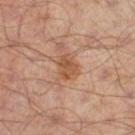Clinical impression:
Imaged during a routine full-body skin examination; the lesion was not biopsied and no histopathology is available.
Acquisition and patient details:
The lesion is located on the right thigh. Automated tile analysis of the lesion measured an area of roughly 5 mm², an outline eccentricity of about 0.7 (0 = round, 1 = elongated), and two-axis asymmetry of about 0.3. And it measured a lesion color around L≈50 a*≈21 b*≈33 in CIELAB, roughly 9 lightness units darker than nearby skin, and a normalized border contrast of about 7.5. The software also gave an automated nevus-likeness rating near 5 out of 100. Cropped from a total-body skin-imaging series; the visible field is about 15 mm. Approximately 3 mm at its widest. This is a cross-polarized tile. The patient is a male aged approximately 65.